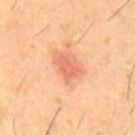Assessment:
The lesion was tiled from a total-body skin photograph and was not biopsied.
Acquisition and patient details:
The lesion is on the front of the torso. A male subject approximately 60 years of age. The total-body-photography lesion software estimated a lesion color around L≈62 a*≈29 b*≈35 in CIELAB, a lesion–skin lightness drop of about 9, and a normalized lesion–skin contrast near 6. It also reported a classifier nevus-likeness of about 45/100. A roughly 15 mm field-of-view crop from a total-body skin photograph. About 3 mm across.